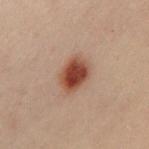Impression:
Part of a total-body skin-imaging series; this lesion was reviewed on a skin check and was not flagged for biopsy.
Clinical summary:
About 4 mm across. On the chest. The patient is a female in their 30s. This is a cross-polarized tile. A 15 mm crop from a total-body photograph taken for skin-cancer surveillance. An algorithmic analysis of the crop reported a footprint of about 9.5 mm², an eccentricity of roughly 0.65, and a symmetry-axis asymmetry near 0.15. The software also gave a detector confidence of about 100 out of 100 that the crop contains a lesion.